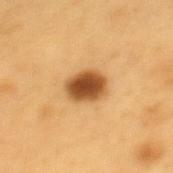Findings:
– follow-up — no biopsy performed (imaged during a skin exam)
– acquisition — ~15 mm tile from a whole-body skin photo
– tile lighting — cross-polarized
– body site — the mid back
– size — about 4 mm
– subject — male, about 60 years old